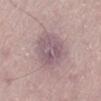notes = imaged on a skin check; not biopsied
TBP lesion metrics = an average lesion color of about L≈55 a*≈17 b*≈13 (CIELAB); lesion-presence confidence of about 85/100
site = the left lower leg
imaging modality = ~15 mm crop, total-body skin-cancer survey
subject = female, aged approximately 60
tile lighting = white-light illumination
lesion size = ≈4.5 mm